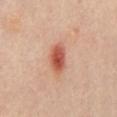Context:
The tile uses cross-polarized illumination. The lesion is on the abdomen. The patient is a male aged approximately 55. A close-up tile cropped from a whole-body skin photograph, about 15 mm across. The recorded lesion diameter is about 4 mm. The lesion-visualizer software estimated an area of roughly 7 mm². The analysis additionally found border irregularity of about 1.5 on a 0–10 scale and a peripheral color-asymmetry measure near 1.5.